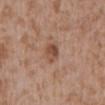Notes:
• follow-up · total-body-photography surveillance lesion; no biopsy
• tile lighting · white-light illumination
• patient · male, approximately 45 years of age
• anatomic site · the front of the torso
• diameter · ≈2.5 mm
• image · ~15 mm tile from a whole-body skin photo